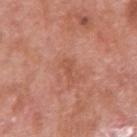Clinical impression:
This lesion was catalogued during total-body skin photography and was not selected for biopsy.
Context:
The lesion is located on the right upper arm. A male patient approximately 70 years of age. Automated image analysis of the tile measured a lesion area of about 2 mm², an outline eccentricity of about 0.95 (0 = round, 1 = elongated), and a shape-asymmetry score of about 0.55 (0 = symmetric). The analysis additionally found border irregularity of about 6 on a 0–10 scale and peripheral color asymmetry of about 0. Imaged with white-light lighting. Cropped from a whole-body photographic skin survey; the tile spans about 15 mm. Measured at roughly 2.5 mm in maximum diameter.